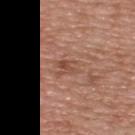Case summary:
• image-analysis metrics — an area of roughly 7 mm², an eccentricity of roughly 0.85, and a shape-asymmetry score of about 0.3 (0 = symmetric); a mean CIELAB color near L≈51 a*≈21 b*≈29 and a normalized lesion–skin contrast near 4.5; border irregularity of about 3 on a 0–10 scale and radial color variation of about 1.5; an automated nevus-likeness rating near 0 out of 100
• patient — male, about 50 years old
• illumination — white-light illumination
• imaging modality — 15 mm crop, total-body photography
• anatomic site — the upper back
• diameter — about 4 mm